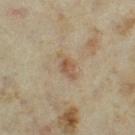– biopsy status — imaged on a skin check; not biopsied
– lesion size — ~3.5 mm (longest diameter)
– lighting — cross-polarized illumination
– anatomic site — the left thigh
– subject — female, aged around 35
– image — 15 mm crop, total-body photography